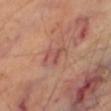| feature | finding |
|---|---|
| workup | imaged on a skin check; not biopsied |
| illumination | cross-polarized |
| acquisition | ~15 mm tile from a whole-body skin photo |
| lesion diameter | ~2.5 mm (longest diameter) |
| body site | the right thigh |
| subject | male, aged around 70 |